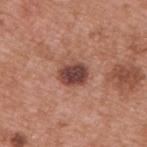Clinical impression: The lesion was tiled from a total-body skin photograph and was not biopsied. Image and clinical context: A male subject in their mid- to late 50s. The total-body-photography lesion software estimated a lesion color around L≈44 a*≈23 b*≈24 in CIELAB, about 15 CIELAB-L* units darker than the surrounding skin, and a normalized lesion–skin contrast near 11. And it measured internal color variation of about 4 on a 0–10 scale and radial color variation of about 1. The tile uses white-light illumination. Approximately 3.5 mm at its widest. A 15 mm close-up tile from a total-body photography series done for melanoma screening. Located on the upper back.